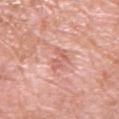Recorded during total-body skin imaging; not selected for excision or biopsy.